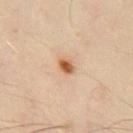<lesion>
<biopsy_status>not biopsied; imaged during a skin examination</biopsy_status>
<lesion_size>
  <long_diameter_mm_approx>2.0</long_diameter_mm_approx>
</lesion_size>
<image>
  <source>total-body photography crop</source>
  <field_of_view_mm>15</field_of_view_mm>
</image>
<lighting>cross-polarized</lighting>
<patient>
  <sex>male</sex>
  <age_approx>45</age_approx>
</patient>
<automated_metrics>
  <border_irregularity_0_10>2.5</border_irregularity_0_10>
  <color_variation_0_10>4.0</color_variation_0_10>
  <peripheral_color_asymmetry>1.5</peripheral_color_asymmetry>
  <nevus_likeness_0_100>100</nevus_likeness_0_100>
  <lesion_detection_confidence_0_100>100</lesion_detection_confidence_0_100>
</automated_metrics>
<site>chest</site>
</lesion>